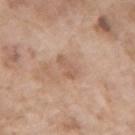Impression:
No biopsy was performed on this lesion — it was imaged during a full skin examination and was not determined to be concerning.
Context:
Imaged with white-light lighting. From the left forearm. The recorded lesion diameter is about 2.5 mm. The lesion-visualizer software estimated a border-irregularity rating of about 2.5/10, a color-variation rating of about 1.5/10, and radial color variation of about 0.5. And it measured an automated nevus-likeness rating near 0 out of 100 and a detector confidence of about 100 out of 100 that the crop contains a lesion. Cropped from a whole-body photographic skin survey; the tile spans about 15 mm. The patient is a female approximately 75 years of age.